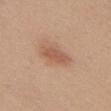- biopsy status: catalogued during a skin exam; not biopsied
- lighting: white-light
- site: the chest
- patient: female, about 45 years old
- imaging modality: ~15 mm tile from a whole-body skin photo